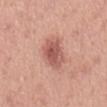Findings:
• location · the mid back
• subject · male, aged 48 to 52
• automated lesion analysis · roughly 12 lightness units darker than nearby skin and a lesion-to-skin contrast of about 8 (normalized; higher = more distinct); an automated nevus-likeness rating near 80 out of 100 and a detector confidence of about 100 out of 100 that the crop contains a lesion
• image source · 15 mm crop, total-body photography
• lighting · white-light illumination
• lesion size · ~4.5 mm (longest diameter)